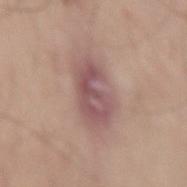Impression: No biopsy was performed on this lesion — it was imaged during a full skin examination and was not determined to be concerning. Clinical summary: Measured at roughly 7 mm in maximum diameter. The lesion is located on the right thigh. Captured under white-light illumination. A lesion tile, about 15 mm wide, cut from a 3D total-body photograph. A male subject, roughly 70 years of age.